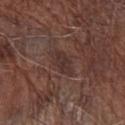notes = catalogued during a skin exam; not biopsied.